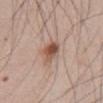Findings:
- notes: imaged on a skin check; not biopsied
- body site: the abdomen
- image: total-body-photography crop, ~15 mm field of view
- subject: male, aged 43–47
- lesion diameter: ≈2.5 mm